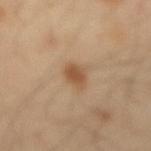The lesion was photographed on a routine skin check and not biopsied; there is no pathology result.
Measured at roughly 2.5 mm in maximum diameter.
The lesion-visualizer software estimated roughly 11 lightness units darker than nearby skin and a normalized lesion–skin contrast near 8. And it measured border irregularity of about 1.5 on a 0–10 scale.
The patient is a male aged around 40.
From the mid back.
This is a cross-polarized tile.
Cropped from a whole-body photographic skin survey; the tile spans about 15 mm.